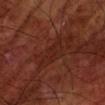Captured during whole-body skin photography for melanoma surveillance; the lesion was not biopsied. This image is a 15 mm lesion crop taken from a total-body photograph. On the left forearm. The total-body-photography lesion software estimated a lesion area of about 6 mm² and two-axis asymmetry of about 0.45. The software also gave an average lesion color of about L≈23 a*≈23 b*≈25 (CIELAB), roughly 4 lightness units darker than nearby skin, and a normalized border contrast of about 5. About 4 mm across. A male subject aged 68–72.